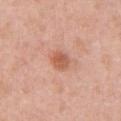biopsy status: catalogued during a skin exam; not biopsied | subject: male, approximately 60 years of age | illumination: white-light | body site: the chest | image source: ~15 mm tile from a whole-body skin photo.